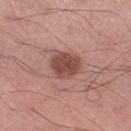notes: total-body-photography surveillance lesion; no biopsy
patient: male, aged approximately 70
body site: the left thigh
image: ~15 mm crop, total-body skin-cancer survey
TBP lesion metrics: a footprint of about 10 mm², an outline eccentricity of about 0.45 (0 = round, 1 = elongated), and two-axis asymmetry of about 0.15; a within-lesion color-variation index near 3/10 and peripheral color asymmetry of about 1